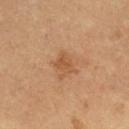{"biopsy_status": "not biopsied; imaged during a skin examination", "lesion_size": {"long_diameter_mm_approx": 2.5}, "site": "right thigh", "image": {"source": "total-body photography crop", "field_of_view_mm": 15}, "patient": {"sex": "male", "age_approx": 60}, "lighting": "cross-polarized"}